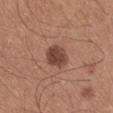Part of a total-body skin-imaging series; this lesion was reviewed on a skin check and was not flagged for biopsy. The lesion is on the left upper arm. Measured at roughly 3.5 mm in maximum diameter. The tile uses white-light illumination. The patient is a male aged approximately 25. A 15 mm close-up extracted from a 3D total-body photography capture.